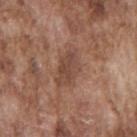Findings:
– follow-up: no biopsy performed (imaged during a skin exam)
– illumination: white-light illumination
– location: the back
– patient: male, aged 73 to 77
– image-analysis metrics: an average lesion color of about L≈46 a*≈20 b*≈27 (CIELAB), a lesion–skin lightness drop of about 8, and a lesion-to-skin contrast of about 6.5 (normalized; higher = more distinct)
– image: 15 mm crop, total-body photography